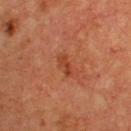Part of a total-body skin-imaging series; this lesion was reviewed on a skin check and was not flagged for biopsy.
The lesion-visualizer software estimated a lesion area of about 3 mm².
This is a cross-polarized tile.
A male patient aged approximately 65.
A 15 mm close-up extracted from a 3D total-body photography capture.
On the chest.